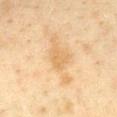{
  "site": "chest",
  "patient": {
    "sex": "female",
    "age_approx": 40
  },
  "image": {
    "source": "total-body photography crop",
    "field_of_view_mm": 15
  }
}